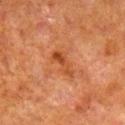Located on the right lower leg. Automated tile analysis of the lesion measured a lesion color around L≈39 a*≈24 b*≈34 in CIELAB and a normalized border contrast of about 6.5. The lesion's longest dimension is about 3.5 mm. A lesion tile, about 15 mm wide, cut from a 3D total-body photograph. A male patient roughly 80 years of age.